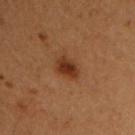  lesion_size:
    long_diameter_mm_approx: 3.0
  lighting: cross-polarized
  site: chest
  automated_metrics:
    border_irregularity_0_10: 2.5
    color_variation_0_10: 3.0
    peripheral_color_asymmetry: 0.5
    nevus_likeness_0_100: 95
    lesion_detection_confidence_0_100: 100
  patient:
    sex: male
    age_approx: 50
  image:
    source: total-body photography crop
    field_of_view_mm: 15